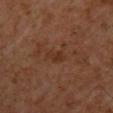Impression: The lesion was tiled from a total-body skin photograph and was not biopsied. Context: The lesion is on the upper back. A male patient, roughly 60 years of age. Longest diameter approximately 2.5 mm. The tile uses cross-polarized illumination. The total-body-photography lesion software estimated a lesion–skin lightness drop of about 5 and a normalized border contrast of about 6. A 15 mm crop from a total-body photograph taken for skin-cancer surveillance.